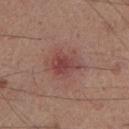notes — no biopsy performed (imaged during a skin exam); tile lighting — cross-polarized illumination; patient — male, approximately 50 years of age; acquisition — total-body-photography crop, ~15 mm field of view; automated metrics — a lesion color around L≈37 a*≈22 b*≈19 in CIELAB, about 8 CIELAB-L* units darker than the surrounding skin, and a normalized lesion–skin contrast near 7; site — the left lower leg.